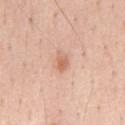{"image": {"source": "total-body photography crop", "field_of_view_mm": 15}, "automated_metrics": {"area_mm2_approx": 3.0, "eccentricity": 0.8, "shape_asymmetry": 0.4, "border_irregularity_0_10": 3.5, "color_variation_0_10": 2.0, "peripheral_color_asymmetry": 0.5, "nevus_likeness_0_100": 45, "lesion_detection_confidence_0_100": 100}, "lighting": "white-light", "site": "chest", "patient": {"sex": "male", "age_approx": 55}}